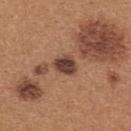Findings:
* biopsy status · no biopsy performed (imaged during a skin exam)
* subject · female, about 40 years old
* size · ≈3 mm
* acquisition · ~15 mm crop, total-body skin-cancer survey
* location · the upper back
* lighting · white-light